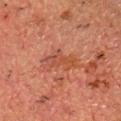A lesion tile, about 15 mm wide, cut from a 3D total-body photograph.
From the chest.
A male patient, aged approximately 80.